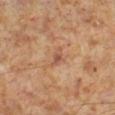<tbp_lesion>
<site>leg</site>
<lesion_size>
  <long_diameter_mm_approx>2.5</long_diameter_mm_approx>
</lesion_size>
<patient>
  <sex>male</sex>
  <age_approx>60</age_approx>
</patient>
<image>
  <source>total-body photography crop</source>
  <field_of_view_mm>15</field_of_view_mm>
</image>
<lighting>cross-polarized</lighting>
<automated_metrics>
  <vs_skin_darker_L>8.0</vs_skin_darker_L>
  <vs_skin_contrast_norm>6.5</vs_skin_contrast_norm>
  <nevus_likeness_0_100>0</nevus_likeness_0_100>
  <lesion_detection_confidence_0_100>100</lesion_detection_confidence_0_100>
</automated_metrics>
</tbp_lesion>